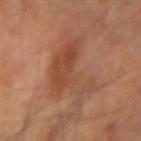  lighting: cross-polarized
  automated_metrics:
    area_mm2_approx: 25.0
    eccentricity: 0.85
    shape_asymmetry: 0.55
    lesion_detection_confidence_0_100: 100
  site: left forearm
  lesion_size:
    long_diameter_mm_approx: 9.0
  image:
    source: total-body photography crop
    field_of_view_mm: 15
  patient:
    sex: male
    age_approx: 65